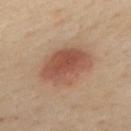biopsy status = no biopsy performed (imaged during a skin exam)
illumination = cross-polarized
automated lesion analysis = a footprint of about 20 mm²; a lesion color around L≈52 a*≈22 b*≈29 in CIELAB, about 11 CIELAB-L* units darker than the surrounding skin, and a normalized border contrast of about 8
diameter = ≈6 mm
location = the upper back
patient = female, roughly 40 years of age
acquisition = ~15 mm tile from a whole-body skin photo